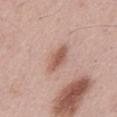This lesion was catalogued during total-body skin photography and was not selected for biopsy.
Captured under white-light illumination.
A region of skin cropped from a whole-body photographic capture, roughly 15 mm wide.
A male subject, roughly 55 years of age.
The lesion is located on the back.
Approximately 3.5 mm at its widest.